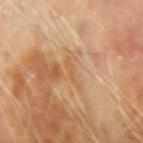No biopsy was performed on this lesion — it was imaged during a full skin examination and was not determined to be concerning. A lesion tile, about 15 mm wide, cut from a 3D total-body photograph. The lesion is located on the left upper arm. A male subject, roughly 70 years of age.